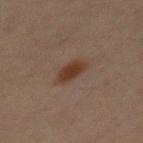The lesion-visualizer software estimated a classifier nevus-likeness of about 100/100 and a lesion-detection confidence of about 100/100. The lesion is located on the mid back. The lesion's longest dimension is about 3 mm. This image is a 15 mm lesion crop taken from a total-body photograph. Captured under cross-polarized illumination. A male patient aged 28–32.